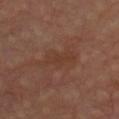Clinical impression: Captured during whole-body skin photography for melanoma surveillance; the lesion was not biopsied. Clinical summary: The subject is a male in their mid-60s. This is a cross-polarized tile. A 15 mm crop from a total-body photograph taken for skin-cancer surveillance. The lesion is located on the front of the torso.